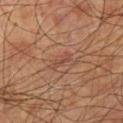Notes:
- automated lesion analysis · an area of roughly 3 mm² and an outline eccentricity of about 0.95 (0 = round, 1 = elongated); border irregularity of about 4 on a 0–10 scale, a within-lesion color-variation index near 0/10, and radial color variation of about 0; a classifier nevus-likeness of about 0/100
- tile lighting · cross-polarized
- body site · the left upper arm
- diameter · about 3 mm
- subject · male, in their mid-70s
- imaging modality · 15 mm crop, total-body photography